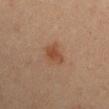Impression: The lesion was tiled from a total-body skin photograph and was not biopsied. Clinical summary: Approximately 3 mm at its widest. A 15 mm crop from a total-body photograph taken for skin-cancer surveillance. Imaged with cross-polarized lighting. The lesion is located on the right upper arm. The patient is a male aged around 60. The lesion-visualizer software estimated a lesion area of about 5 mm², an outline eccentricity of about 0.75 (0 = round, 1 = elongated), and two-axis asymmetry of about 0.35. It also reported a mean CIELAB color near L≈38 a*≈19 b*≈27 and a normalized lesion–skin contrast near 7. It also reported an automated nevus-likeness rating near 95 out of 100 and lesion-presence confidence of about 100/100.